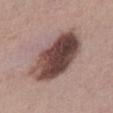notes — imaged on a skin check; not biopsied
tile lighting — white-light
location — the abdomen
imaging modality — 15 mm crop, total-body photography
automated lesion analysis — roughly 18 lightness units darker than nearby skin and a lesion-to-skin contrast of about 13 (normalized; higher = more distinct); a border-irregularity rating of about 2/10 and a within-lesion color-variation index near 9/10
lesion size — ≈8 mm
subject — female, aged approximately 40